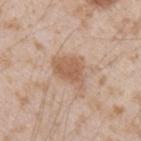Assessment:
Captured during whole-body skin photography for melanoma surveillance; the lesion was not biopsied.
Clinical summary:
Cropped from a total-body skin-imaging series; the visible field is about 15 mm. Approximately 4.5 mm at its widest. From the left forearm. The patient is a male roughly 25 years of age. The total-body-photography lesion software estimated a lesion color around L≈59 a*≈19 b*≈31 in CIELAB, about 10 CIELAB-L* units darker than the surrounding skin, and a normalized border contrast of about 7. Imaged with white-light lighting.